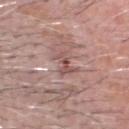biopsy status: imaged on a skin check; not biopsied
lesion diameter: ≈2.5 mm
site: the head or neck
acquisition: ~15 mm crop, total-body skin-cancer survey
patient: male, about 60 years old
illumination: white-light